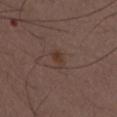Q: Was a biopsy performed?
A: imaged on a skin check; not biopsied
Q: Lesion size?
A: ≈2.5 mm
Q: What kind of image is this?
A: ~15 mm tile from a whole-body skin photo
Q: Lesion location?
A: the abdomen
Q: What are the patient's age and sex?
A: male, aged 48–52
Q: How was the tile lit?
A: white-light illumination
Q: What did automated image analysis measure?
A: a lesion area of about 3 mm², a shape eccentricity near 0.85, and two-axis asymmetry of about 0.3; a border-irregularity index near 3/10, a within-lesion color-variation index near 1/10, and radial color variation of about 0.5; a classifier nevus-likeness of about 40/100